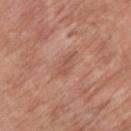Captured during whole-body skin photography for melanoma surveillance; the lesion was not biopsied.
Automated image analysis of the tile measured an eccentricity of roughly 0.85 and a shape-asymmetry score of about 0.3 (0 = symmetric). And it measured a lesion–skin lightness drop of about 7 and a normalized lesion–skin contrast near 5. The analysis additionally found border irregularity of about 3 on a 0–10 scale, a within-lesion color-variation index near 1.5/10, and radial color variation of about 0.5.
The tile uses white-light illumination.
A 15 mm close-up tile from a total-body photography series done for melanoma screening.
A male patient aged around 65.
On the upper back.